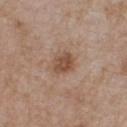Findings:
• workup — catalogued during a skin exam; not biopsied
• size — ≈3 mm
• subject — male, aged 63 to 67
• acquisition — ~15 mm tile from a whole-body skin photo
• tile lighting — white-light illumination
• location — the front of the torso